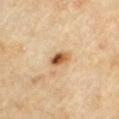A male patient approximately 70 years of age.
A region of skin cropped from a whole-body photographic capture, roughly 15 mm wide.
The lesion is on the chest.